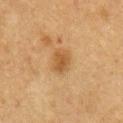lesion size = about 3 mm; imaging modality = total-body-photography crop, ~15 mm field of view; patient = male, aged around 75; site = the chest.